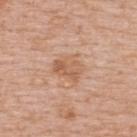Assessment:
Recorded during total-body skin imaging; not selected for excision or biopsy.
Clinical summary:
On the upper back. A 15 mm close-up extracted from a 3D total-body photography capture. Measured at roughly 3.5 mm in maximum diameter. Captured under white-light illumination. A female subject roughly 65 years of age. An algorithmic analysis of the crop reported an outline eccentricity of about 0.55 (0 = round, 1 = elongated) and a symmetry-axis asymmetry near 0.25. It also reported a lesion color around L≈60 a*≈21 b*≈33 in CIELAB, about 8 CIELAB-L* units darker than the surrounding skin, and a normalized lesion–skin contrast near 5.5.